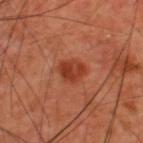Assessment: Recorded during total-body skin imaging; not selected for excision or biopsy. Clinical summary: The tile uses cross-polarized illumination. Measured at roughly 3 mm in maximum diameter. On the upper back. A close-up tile cropped from a whole-body skin photograph, about 15 mm across. A male patient, about 60 years old.